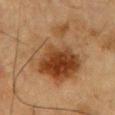workup = total-body-photography surveillance lesion; no biopsy | lesion diameter = ~8.5 mm (longest diameter) | TBP lesion metrics = a footprint of about 34 mm² and two-axis asymmetry of about 0.45; a mean CIELAB color near L≈38 a*≈19 b*≈32, a lesion–skin lightness drop of about 11, and a normalized border contrast of about 9.5; a nevus-likeness score of about 100/100 and a lesion-detection confidence of about 100/100 | anatomic site = the chest | tile lighting = cross-polarized illumination | patient = male, about 85 years old or older | acquisition = total-body-photography crop, ~15 mm field of view.